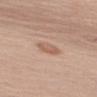No biopsy was performed on this lesion — it was imaged during a full skin examination and was not determined to be concerning. The subject is a male aged approximately 60. A 15 mm close-up extracted from a 3D total-body photography capture. The tile uses white-light illumination. The recorded lesion diameter is about 3 mm. The lesion-visualizer software estimated a border-irregularity index near 1.5/10, internal color variation of about 2.5 on a 0–10 scale, and a peripheral color-asymmetry measure near 0.5. The analysis additionally found a nevus-likeness score of about 20/100 and a detector confidence of about 100 out of 100 that the crop contains a lesion. The lesion is on the arm.